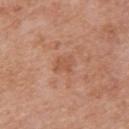Recorded during total-body skin imaging; not selected for excision or biopsy. The lesion's longest dimension is about 2.5 mm. A close-up tile cropped from a whole-body skin photograph, about 15 mm across. A female patient aged 38 to 42. On the back.